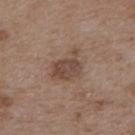workup = total-body-photography surveillance lesion; no biopsy
lesion diameter = about 4.5 mm
lighting = white-light illumination
automated lesion analysis = a lesion area of about 8.5 mm², a shape eccentricity near 0.7, and two-axis asymmetry of about 0.4
anatomic site = the upper back
image = ~15 mm crop, total-body skin-cancer survey
subject = male, in their 50s